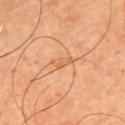Notes:
• follow-up · total-body-photography surveillance lesion; no biopsy
• lighting · cross-polarized
• imaging modality · total-body-photography crop, ~15 mm field of view
• patient · male, approximately 65 years of age
• site · the left upper arm